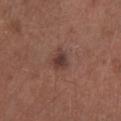Q: Was this lesion biopsied?
A: catalogued during a skin exam; not biopsied
Q: What are the patient's age and sex?
A: male, aged around 70
Q: What is the anatomic site?
A: the left lower leg
Q: What lighting was used for the tile?
A: white-light illumination
Q: What kind of image is this?
A: 15 mm crop, total-body photography